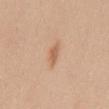<record>
<biopsy_status>not biopsied; imaged during a skin examination</biopsy_status>
<image>
  <source>total-body photography crop</source>
  <field_of_view_mm>15</field_of_view_mm>
</image>
<lighting>white-light</lighting>
<site>mid back</site>
<patient>
  <sex>female</sex>
  <age_approx>30</age_approx>
</patient>
<lesion_size>
  <long_diameter_mm_approx>3.0</long_diameter_mm_approx>
</lesion_size>
<automated_metrics>
  <border_irregularity_0_10>3.0</border_irregularity_0_10>
  <color_variation_0_10>2.0</color_variation_0_10>
  <peripheral_color_asymmetry>0.5</peripheral_color_asymmetry>
  <lesion_detection_confidence_0_100>100</lesion_detection_confidence_0_100>
</automated_metrics>
</record>